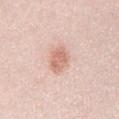A 15 mm close-up extracted from a 3D total-body photography capture.
This is a white-light tile.
A male subject, roughly 25 years of age.
Longest diameter approximately 4 mm.
The lesion is located on the chest.
The total-body-photography lesion software estimated a border-irregularity index near 2/10, a color-variation rating of about 2.5/10, and radial color variation of about 1. The analysis additionally found an automated nevus-likeness rating near 70 out of 100 and lesion-presence confidence of about 100/100.